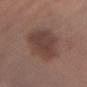notes — imaged on a skin check; not biopsied
image-analysis metrics — an area of roughly 21 mm²
acquisition — ~15 mm crop, total-body skin-cancer survey
body site — the left forearm
subject — male, aged 53 to 57
diameter — ~5.5 mm (longest diameter)